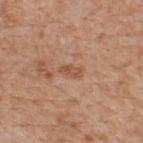biopsy status: total-body-photography surveillance lesion; no biopsy | location: the upper back | subject: male, roughly 60 years of age | size: ≈2.5 mm | acquisition: ~15 mm crop, total-body skin-cancer survey | lighting: white-light | automated lesion analysis: a footprint of about 3 mm², an outline eccentricity of about 0.9 (0 = round, 1 = elongated), and a shape-asymmetry score of about 0.35 (0 = symmetric).